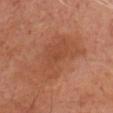No biopsy was performed on this lesion — it was imaged during a full skin examination and was not determined to be concerning.
This is a cross-polarized tile.
From the head or neck.
Approximately 8 mm at its widest.
The patient is a male roughly 70 years of age.
A region of skin cropped from a whole-body photographic capture, roughly 15 mm wide.